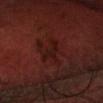workup = imaged on a skin check; not biopsied | subject = male, aged 68 to 72 | tile lighting = cross-polarized illumination | imaging modality = ~15 mm crop, total-body skin-cancer survey | site = the head or neck.